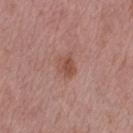Captured during whole-body skin photography for melanoma surveillance; the lesion was not biopsied.
A 15 mm close-up extracted from a 3D total-body photography capture.
This is a white-light tile.
The lesion's longest dimension is about 3 mm.
The lesion is on the arm.
A male subject, aged around 55.